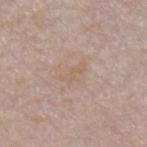Findings:
* notes · no biopsy performed (imaged during a skin exam)
* subject · male, aged 53 to 57
* location · the abdomen
* image · ~15 mm tile from a whole-body skin photo